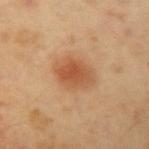This lesion was catalogued during total-body skin photography and was not selected for biopsy. Automated image analysis of the tile measured a mean CIELAB color near L≈53 a*≈23 b*≈37, a lesion–skin lightness drop of about 10, and a normalized lesion–skin contrast near 7.5. And it measured a nevus-likeness score of about 100/100 and lesion-presence confidence of about 100/100. The tile uses cross-polarized illumination. About 4 mm across. Located on the left upper arm. A male subject, approximately 50 years of age. A 15 mm close-up tile from a total-body photography series done for melanoma screening.